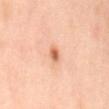Q: Was a biopsy performed?
A: imaged on a skin check; not biopsied
Q: Who is the patient?
A: female, aged 38–42
Q: What lighting was used for the tile?
A: cross-polarized illumination
Q: Where on the body is the lesion?
A: the mid back
Q: Automated lesion metrics?
A: a lesion area of about 2.5 mm² and two-axis asymmetry of about 0.3; a normalized lesion–skin contrast near 8.5; an automated nevus-likeness rating near 95 out of 100 and lesion-presence confidence of about 100/100
Q: What is the imaging modality?
A: 15 mm crop, total-body photography
Q: How large is the lesion?
A: ≈2 mm